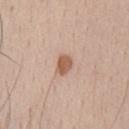Part of a total-body skin-imaging series; this lesion was reviewed on a skin check and was not flagged for biopsy. Imaged with white-light lighting. A male subject in their mid-30s. Located on the chest. Cropped from a total-body skin-imaging series; the visible field is about 15 mm. About 2.5 mm across.